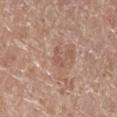  patient:
    sex: female
    age_approx: 75
  site: left lower leg
  automated_metrics:
    area_mm2_approx: 2.5
    eccentricity: 0.85
    border_irregularity_0_10: 5.5
    color_variation_0_10: 0.0
    lesion_detection_confidence_0_100: 100
  image:
    source: total-body photography crop
    field_of_view_mm: 15